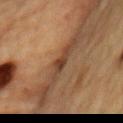Recorded during total-body skin imaging; not selected for excision or biopsy.
An algorithmic analysis of the crop reported a lesion-to-skin contrast of about 8 (normalized; higher = more distinct). It also reported a nevus-likeness score of about 20/100 and lesion-presence confidence of about 90/100.
About 3 mm across.
Imaged with cross-polarized lighting.
The patient is a male approximately 85 years of age or older.
From the chest.
Cropped from a total-body skin-imaging series; the visible field is about 15 mm.